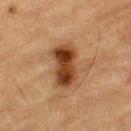follow-up: total-body-photography surveillance lesion; no biopsy
acquisition: ~15 mm crop, total-body skin-cancer survey
subject: male, aged 83 or older
lighting: cross-polarized illumination
body site: the left thigh
diameter: about 5 mm